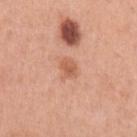Impression:
No biopsy was performed on this lesion — it was imaged during a full skin examination and was not determined to be concerning.
Clinical summary:
The total-body-photography lesion software estimated a footprint of about 3.5 mm² and an eccentricity of roughly 0.7. It also reported about 9 CIELAB-L* units darker than the surrounding skin and a lesion-to-skin contrast of about 6.5 (normalized; higher = more distinct). And it measured a within-lesion color-variation index near 2.5/10 and a peripheral color-asymmetry measure near 1. It also reported an automated nevus-likeness rating near 50 out of 100 and a lesion-detection confidence of about 100/100. A lesion tile, about 15 mm wide, cut from a 3D total-body photograph. The subject is a female aged approximately 40. The lesion's longest dimension is about 2.5 mm. This is a white-light tile. On the arm.